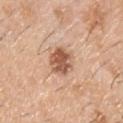workup: catalogued during a skin exam; not biopsied | imaging modality: ~15 mm tile from a whole-body skin photo | diameter: about 3.5 mm | tile lighting: white-light | automated lesion analysis: a shape eccentricity near 0.55; a lesion color around L≈57 a*≈22 b*≈32 in CIELAB, a lesion–skin lightness drop of about 14, and a lesion-to-skin contrast of about 9 (normalized; higher = more distinct); a border-irregularity rating of about 2/10, internal color variation of about 5 on a 0–10 scale, and a peripheral color-asymmetry measure near 1.5; lesion-presence confidence of about 100/100 | patient: male, aged 58–62 | site: the chest.